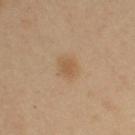No biopsy was performed on this lesion — it was imaged during a full skin examination and was not determined to be concerning.
This is a cross-polarized tile.
A 15 mm crop from a total-body photograph taken for skin-cancer surveillance.
Longest diameter approximately 2.5 mm.
The lesion is on the left arm.
A female subject, about 40 years old.